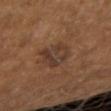This lesion was catalogued during total-body skin photography and was not selected for biopsy.
A roughly 15 mm field-of-view crop from a total-body skin photograph.
Longest diameter approximately 4 mm.
On the right forearm.
The total-body-photography lesion software estimated a footprint of about 11 mm², an eccentricity of roughly 0.6, and two-axis asymmetry of about 0.3. And it measured roughly 7 lightness units darker than nearby skin and a lesion-to-skin contrast of about 7.5 (normalized; higher = more distinct). It also reported a peripheral color-asymmetry measure near 1.5.
A male patient, aged 58–62.
Captured under cross-polarized illumination.